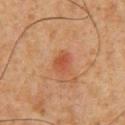A male subject aged 58 to 62. From the chest. Cropped from a whole-body photographic skin survey; the tile spans about 15 mm.